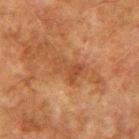Q: Illumination type?
A: cross-polarized illumination
Q: Patient demographics?
A: male, approximately 75 years of age
Q: How large is the lesion?
A: ≈4 mm
Q: What did automated image analysis measure?
A: a shape eccentricity near 0.8; a lesion color around L≈41 a*≈22 b*≈32 in CIELAB, roughly 7 lightness units darker than nearby skin, and a normalized lesion–skin contrast near 5.5; a nevus-likeness score of about 0/100 and a detector confidence of about 100 out of 100 that the crop contains a lesion
Q: What is the anatomic site?
A: the right upper arm
Q: How was this image acquired?
A: 15 mm crop, total-body photography